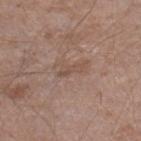<record>
  <site>leg</site>
  <image>
    <source>total-body photography crop</source>
    <field_of_view_mm>15</field_of_view_mm>
  </image>
  <patient>
    <sex>male</sex>
    <age_approx>45</age_approx>
  </patient>
</record>